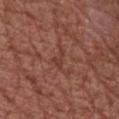{
  "site": "chest",
  "lesion_size": {
    "long_diameter_mm_approx": 3.0
  },
  "automated_metrics": {
    "area_mm2_approx": 3.0,
    "eccentricity": 0.85,
    "shape_asymmetry": 0.55
  },
  "image": {
    "source": "total-body photography crop",
    "field_of_view_mm": 15
  },
  "patient": {
    "sex": "male",
    "age_approx": 75
  }
}